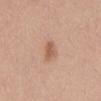Clinical summary: The subject is a female approximately 55 years of age. A lesion tile, about 15 mm wide, cut from a 3D total-body photograph. Located on the front of the torso.